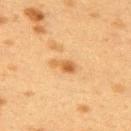The lesion was tiled from a total-body skin photograph and was not biopsied.